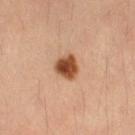The lesion was tiled from a total-body skin photograph and was not biopsied. A female subject about 35 years old. The recorded lesion diameter is about 3 mm. A close-up tile cropped from a whole-body skin photograph, about 15 mm across. The total-body-photography lesion software estimated an average lesion color of about L≈49 a*≈25 b*≈36 (CIELAB) and a lesion–skin lightness drop of about 18. And it measured an automated nevus-likeness rating near 100 out of 100 and a lesion-detection confidence of about 100/100. From the right thigh. Captured under cross-polarized illumination.